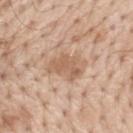follow-up: no biopsy performed (imaged during a skin exam)
imaging modality: ~15 mm crop, total-body skin-cancer survey
illumination: white-light
automated metrics: a lesion–skin lightness drop of about 9 and a normalized border contrast of about 6; internal color variation of about 3 on a 0–10 scale; an automated nevus-likeness rating near 0 out of 100
body site: the mid back
lesion size: ~4.5 mm (longest diameter)
subject: male, aged 68–72